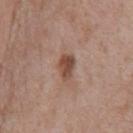Assessment:
Recorded during total-body skin imaging; not selected for excision or biopsy.
Background:
Captured under white-light illumination. This image is a 15 mm lesion crop taken from a total-body photograph. The total-body-photography lesion software estimated a shape eccentricity near 0.8 and two-axis asymmetry of about 0.25. The analysis additionally found an average lesion color of about L≈47 a*≈20 b*≈27 (CIELAB). The lesion is on the chest. The recorded lesion diameter is about 3.5 mm. The patient is a male approximately 70 years of age.